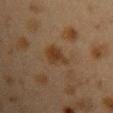The lesion was photographed on a routine skin check and not biopsied; there is no pathology result. The lesion is located on the front of the torso. The patient is a female aged 38 to 42. A close-up tile cropped from a whole-body skin photograph, about 15 mm across.